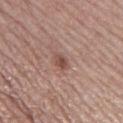biopsy status: no biopsy performed (imaged during a skin exam)
anatomic site: the left thigh
imaging modality: 15 mm crop, total-body photography
image-analysis metrics: a lesion area of about 3 mm² and a shape-asymmetry score of about 0.25 (0 = symmetric); a nevus-likeness score of about 75/100
tile lighting: white-light illumination
patient: female, aged 58–62
diameter: ≈2 mm